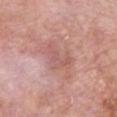Impression:
The lesion was tiled from a total-body skin photograph and was not biopsied.
Acquisition and patient details:
The recorded lesion diameter is about 5.5 mm. The patient is a male aged 78–82. The lesion is on the chest. Automated tile analysis of the lesion measured a lesion area of about 12 mm², an outline eccentricity of about 0.85 (0 = round, 1 = elongated), and two-axis asymmetry of about 0.4. The analysis additionally found a lesion color around L≈59 a*≈24 b*≈24 in CIELAB and a lesion–skin lightness drop of about 7. A 15 mm close-up tile from a total-body photography series done for melanoma screening. Imaged with white-light lighting.